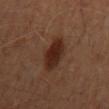notes = no biopsy performed (imaged during a skin exam) | image source = ~15 mm crop, total-body skin-cancer survey | body site = the abdomen | subject = male, about 60 years old.